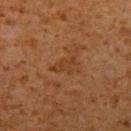follow-up: no biopsy performed (imaged during a skin exam)
image source: 15 mm crop, total-body photography
tile lighting: cross-polarized illumination
site: the left upper arm
subject: male, aged around 60
size: ≈3.5 mm